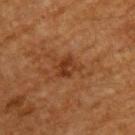Recorded during total-body skin imaging; not selected for excision or biopsy. On the upper back. A male subject, in their 60s. A 15 mm close-up tile from a total-body photography series done for melanoma screening.